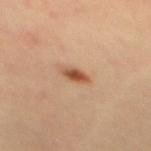notes=no biopsy performed (imaged during a skin exam)
automated lesion analysis=a nevus-likeness score of about 100/100
lighting=cross-polarized
diameter=~3 mm (longest diameter)
site=the upper back
subject=female, aged approximately 35
imaging modality=~15 mm crop, total-body skin-cancer survey